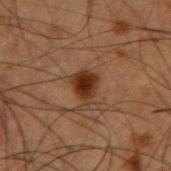Background:
A male patient about 50 years old. A 15 mm close-up extracted from a 3D total-body photography capture. Imaged with cross-polarized lighting. Longest diameter approximately 3.5 mm. From the right forearm.